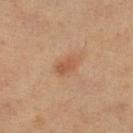The patient is a female aged around 70. Measured at roughly 2.5 mm in maximum diameter. Imaged with cross-polarized lighting. Automated tile analysis of the lesion measured an area of roughly 3 mm² and a shape-asymmetry score of about 0.25 (0 = symmetric). The software also gave a lesion-to-skin contrast of about 6 (normalized; higher = more distinct). The software also gave a nevus-likeness score of about 75/100 and lesion-presence confidence of about 100/100. On the left lower leg. This image is a 15 mm lesion crop taken from a total-body photograph.